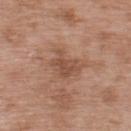Assessment: No biopsy was performed on this lesion — it was imaged during a full skin examination and was not determined to be concerning. Image and clinical context: Approximately 3.5 mm at its widest. Cropped from a total-body skin-imaging series; the visible field is about 15 mm. From the upper back. Captured under white-light illumination. The subject is a male aged around 50.